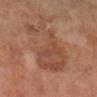Imaged during a routine full-body skin examination; the lesion was not biopsied and no histopathology is available. A male patient aged around 65. Cropped from a whole-body photographic skin survey; the tile spans about 15 mm. From the left leg.